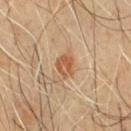Recorded during total-body skin imaging; not selected for excision or biopsy. Imaged with cross-polarized lighting. A male subject roughly 55 years of age. A 15 mm crop from a total-body photograph taken for skin-cancer surveillance. About 3 mm across. Located on the front of the torso.